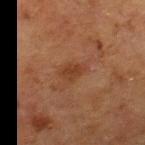The lesion was tiled from a total-body skin photograph and was not biopsied. A lesion tile, about 15 mm wide, cut from a 3D total-body photograph. The subject is a female aged approximately 50. Located on the left thigh.